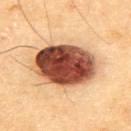biopsy_status: not biopsied; imaged during a skin examination
automated_metrics:
  color_variation_0_10: 10.0
patient:
  sex: male
  age_approx: 70
image:
  source: total-body photography crop
  field_of_view_mm: 15
site: upper back
lighting: cross-polarized
lesion_size:
  long_diameter_mm_approx: 7.5